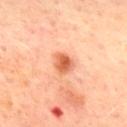The lesion was tiled from a total-body skin photograph and was not biopsied.
Located on the chest.
An algorithmic analysis of the crop reported a footprint of about 5.5 mm² and a shape eccentricity near 0.45. And it measured an automated nevus-likeness rating near 95 out of 100.
Cropped from a whole-body photographic skin survey; the tile spans about 15 mm.
This is a cross-polarized tile.
Longest diameter approximately 2.5 mm.
A male patient aged 68–72.